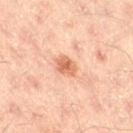Impression:
Imaged during a routine full-body skin examination; the lesion was not biopsied and no histopathology is available.
Acquisition and patient details:
The lesion's longest dimension is about 2.5 mm. The tile uses cross-polarized illumination. A 15 mm close-up tile from a total-body photography series done for melanoma screening. An algorithmic analysis of the crop reported a border-irregularity index near 2.5/10 and internal color variation of about 2.5 on a 0–10 scale. The lesion is on the right thigh. The patient is a male aged 43–47.